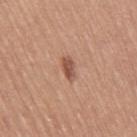- biopsy status · total-body-photography surveillance lesion; no biopsy
- patient · female, in their mid- to late 50s
- body site · the leg
- size · about 3 mm
- image · 15 mm crop, total-body photography
- illumination · white-light illumination